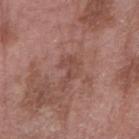Impression: Captured during whole-body skin photography for melanoma surveillance; the lesion was not biopsied.